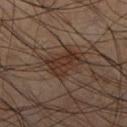Impression:
The lesion was tiled from a total-body skin photograph and was not biopsied.
Clinical summary:
The lesion is located on the leg. A male subject, about 60 years old. A lesion tile, about 15 mm wide, cut from a 3D total-body photograph. This is a cross-polarized tile. About 4.5 mm across.